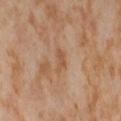A female patient, aged approximately 55.
This is a cross-polarized tile.
Approximately 3 mm at its widest.
This image is a 15 mm lesion crop taken from a total-body photograph.
The lesion is on the right thigh.